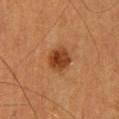No biopsy was performed on this lesion — it was imaged during a full skin examination and was not determined to be concerning. From the upper back. The recorded lesion diameter is about 3.5 mm. The tile uses cross-polarized illumination. A region of skin cropped from a whole-body photographic capture, roughly 15 mm wide. A female subject aged 58–62.